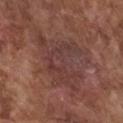Recorded during total-body skin imaging; not selected for excision or biopsy.